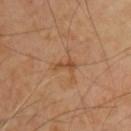Acquisition and patient details: This is a cross-polarized tile. A 15 mm close-up extracted from a 3D total-body photography capture. The patient is a male aged around 60. The lesion is on the chest.